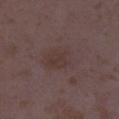Captured during whole-body skin photography for melanoma surveillance; the lesion was not biopsied.
A female patient aged approximately 35.
The recorded lesion diameter is about 5 mm.
Cropped from a total-body skin-imaging series; the visible field is about 15 mm.
From the leg.
Imaged with white-light lighting.